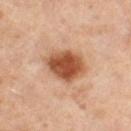This lesion was catalogued during total-body skin photography and was not selected for biopsy. The subject is a female in their 50s. The total-body-photography lesion software estimated an outline eccentricity of about 0.35 (0 = round, 1 = elongated) and a symmetry-axis asymmetry near 0.2. The software also gave a nevus-likeness score of about 100/100 and a detector confidence of about 100 out of 100 that the crop contains a lesion. A 15 mm close-up tile from a total-body photography series done for melanoma screening. Located on the right thigh. The tile uses cross-polarized illumination.